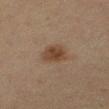biopsy status=catalogued during a skin exam; not biopsied | site=the leg | subject=female, roughly 30 years of age | lesion diameter=~3.5 mm (longest diameter) | tile lighting=cross-polarized | image source=~15 mm tile from a whole-body skin photo.